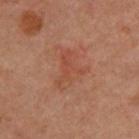- biopsy status — imaged on a skin check; not biopsied
- anatomic site — the upper back
- image source — total-body-photography crop, ~15 mm field of view
- subject — female, aged around 45
- diameter — about 4.5 mm
- automated metrics — an average lesion color of about L≈48 a*≈25 b*≈31 (CIELAB), about 6 CIELAB-L* units darker than the surrounding skin, and a lesion-to-skin contrast of about 4.5 (normalized; higher = more distinct)